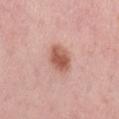The lesion was tiled from a total-body skin photograph and was not biopsied.
This is a white-light tile.
On the left thigh.
An algorithmic analysis of the crop reported an area of roughly 8 mm², an eccentricity of roughly 0.5, and a symmetry-axis asymmetry near 0.1. The software also gave a lesion color around L≈58 a*≈24 b*≈28 in CIELAB, roughly 13 lightness units darker than nearby skin, and a normalized lesion–skin contrast near 8.5. The analysis additionally found peripheral color asymmetry of about 1.
Cropped from a whole-body photographic skin survey; the tile spans about 15 mm.
A female patient, about 45 years old.
Longest diameter approximately 3.5 mm.